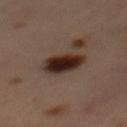{"biopsy_status": "not biopsied; imaged during a skin examination", "automated_metrics": {"area_mm2_approx": 10.0, "eccentricity": 0.75, "shape_asymmetry": 0.2}, "patient": {"sex": "female", "age_approx": 45}, "site": "mid back", "image": {"source": "total-body photography crop", "field_of_view_mm": 15}}